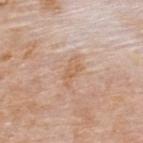| feature | finding |
|---|---|
| notes | no biopsy performed (imaged during a skin exam) |
| patient | male, approximately 75 years of age |
| image source | 15 mm crop, total-body photography |
| automated metrics | an area of roughly 4 mm²; a nevus-likeness score of about 0/100 and a lesion-detection confidence of about 100/100 |
| site | the upper back |